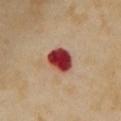lesion diameter: about 3.5 mm; subject: female, aged approximately 55; anatomic site: the chest; image source: 15 mm crop, total-body photography; tile lighting: cross-polarized.